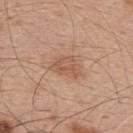Assessment:
The lesion was photographed on a routine skin check and not biopsied; there is no pathology result.
Context:
A male patient, about 50 years old. Cropped from a total-body skin-imaging series; the visible field is about 15 mm. The lesion is on the back. The tile uses white-light illumination. Approximately 3.5 mm at its widest.